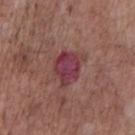Impression: Part of a total-body skin-imaging series; this lesion was reviewed on a skin check and was not flagged for biopsy. Image and clinical context: A male subject, in their 70s. The recorded lesion diameter is about 4.5 mm. Captured under white-light illumination. On the chest. The total-body-photography lesion software estimated an area of roughly 10 mm² and a symmetry-axis asymmetry near 0.25. A 15 mm close-up extracted from a 3D total-body photography capture.